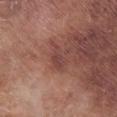illumination=white-light illumination
image source=~15 mm tile from a whole-body skin photo
diameter=~3 mm (longest diameter)
site=the right lower leg
subject=male, aged 73 to 77